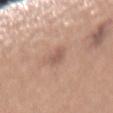Impression: The lesion was photographed on a routine skin check and not biopsied; there is no pathology result. Image and clinical context: This image is a 15 mm lesion crop taken from a total-body photograph. Imaged with white-light lighting. A female patient aged around 40. An algorithmic analysis of the crop reported a nevus-likeness score of about 0/100 and a detector confidence of about 100 out of 100 that the crop contains a lesion. The lesion is on the mid back.